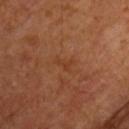{
  "biopsy_status": "not biopsied; imaged during a skin examination",
  "site": "chest",
  "image": {
    "source": "total-body photography crop",
    "field_of_view_mm": 15
  },
  "patient": {
    "sex": "male",
    "age_approx": 65
  },
  "lighting": "cross-polarized"
}